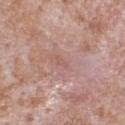Recorded during total-body skin imaging; not selected for excision or biopsy.
The subject is a male approximately 65 years of age.
This is a white-light tile.
A 15 mm close-up tile from a total-body photography series done for melanoma screening.
Located on the front of the torso.